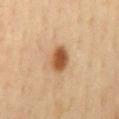Acquisition and patient details:
This is a cross-polarized tile. An algorithmic analysis of the crop reported an area of roughly 6.5 mm² and two-axis asymmetry of about 0.3. A male patient aged 53–57. The recorded lesion diameter is about 3.5 mm. From the mid back. A 15 mm crop from a total-body photograph taken for skin-cancer surveillance.